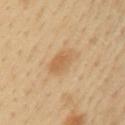  biopsy_status: not biopsied; imaged during a skin examination
  lighting: cross-polarized
  image:
    source: total-body photography crop
    field_of_view_mm: 15
  lesion_size:
    long_diameter_mm_approx: 5.0
  patient:
    sex: male
    age_approx: 40
  site: arm